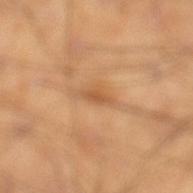Recorded during total-body skin imaging; not selected for excision or biopsy.
Located on the left lower leg.
A 15 mm close-up extracted from a 3D total-body photography capture.
A male subject aged 38 to 42.
Captured under cross-polarized illumination.
Approximately 3 mm at its widest.
The total-body-photography lesion software estimated an area of roughly 2.5 mm² and a symmetry-axis asymmetry near 0.15. And it measured a border-irregularity index near 2.5/10, a color-variation rating of about 0/10, and peripheral color asymmetry of about 0. The software also gave lesion-presence confidence of about 95/100.